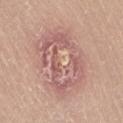Captured during whole-body skin photography for melanoma surveillance; the lesion was not biopsied. A region of skin cropped from a whole-body photographic capture, roughly 15 mm wide. This is a white-light tile. Approximately 7.5 mm at its widest. Automated image analysis of the tile measured a lesion color around L≈60 a*≈21 b*≈24 in CIELAB, roughly 8 lightness units darker than nearby skin, and a lesion-to-skin contrast of about 5.5 (normalized; higher = more distinct). The analysis additionally found an automated nevus-likeness rating near 0 out of 100 and a detector confidence of about 95 out of 100 that the crop contains a lesion. Located on the lower back. The subject is a male aged around 65.